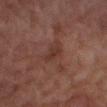Part of a total-body skin-imaging series; this lesion was reviewed on a skin check and was not flagged for biopsy. Imaged with cross-polarized lighting. A region of skin cropped from a whole-body photographic capture, roughly 15 mm wide. The patient is a female about 60 years old. Located on the leg. The recorded lesion diameter is about 3 mm.